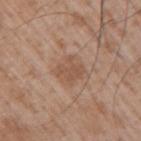– notes: catalogued during a skin exam; not biopsied
– image: total-body-photography crop, ~15 mm field of view
– lesion diameter: about 3 mm
– patient: male, aged 48 to 52
– anatomic site: the right upper arm
– lighting: white-light illumination
– automated lesion analysis: an average lesion color of about L≈52 a*≈19 b*≈30 (CIELAB) and roughly 7 lightness units darker than nearby skin; border irregularity of about 4.5 on a 0–10 scale, internal color variation of about 1.5 on a 0–10 scale, and radial color variation of about 0.5